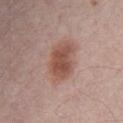No biopsy was performed on this lesion — it was imaged during a full skin examination and was not determined to be concerning. The lesion-visualizer software estimated a footprint of about 16 mm². The software also gave a border-irregularity index near 2/10, internal color variation of about 4 on a 0–10 scale, and radial color variation of about 1. This image is a 15 mm lesion crop taken from a total-body photograph. The subject is a male aged 53–57. The lesion is located on the abdomen. The recorded lesion diameter is about 6 mm. Imaged with white-light lighting.